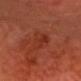- follow-up — total-body-photography surveillance lesion; no biopsy
- body site — the head or neck
- size — ~6 mm (longest diameter)
- subject — male, aged approximately 65
- image source — ~15 mm crop, total-body skin-cancer survey